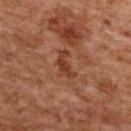Recorded during total-body skin imaging; not selected for excision or biopsy. The total-body-photography lesion software estimated border irregularity of about 5.5 on a 0–10 scale and a peripheral color-asymmetry measure near 0.5. It also reported a classifier nevus-likeness of about 0/100 and a lesion-detection confidence of about 100/100. Measured at roughly 3.5 mm in maximum diameter. Captured under cross-polarized illumination. This image is a 15 mm lesion crop taken from a total-body photograph. A female subject aged around 60. The lesion is on the upper back.